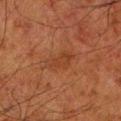biopsy_status: not biopsied; imaged during a skin examination
lesion_size:
  long_diameter_mm_approx: 4.0
site: left lower leg
patient:
  sex: male
  age_approx: 80
automated_metrics:
  area_mm2_approx: 6.5
  shape_asymmetry: 0.3
  nevus_likeness_0_100: 0
  lesion_detection_confidence_0_100: 100
image:
  source: total-body photography crop
  field_of_view_mm: 15